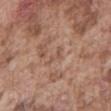The subject is a male aged approximately 75.
The total-body-photography lesion software estimated a border-irregularity index near 6.5/10, a color-variation rating of about 0/10, and a peripheral color-asymmetry measure near 0.
About 2.5 mm across.
On the front of the torso.
A close-up tile cropped from a whole-body skin photograph, about 15 mm across.